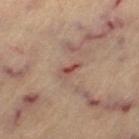| field | value |
|---|---|
| image source | ~15 mm crop, total-body skin-cancer survey |
| automated metrics | about 9 CIELAB-L* units darker than the surrounding skin and a normalized border contrast of about 7.5; a border-irregularity rating of about 3/10 and radial color variation of about 0; a detector confidence of about 100 out of 100 that the crop contains a lesion |
| location | the left thigh |
| diameter | ~2.5 mm (longest diameter) |
| patient | female, aged 63–67 |
| lighting | cross-polarized |